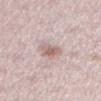Q: Was this lesion biopsied?
A: imaged on a skin check; not biopsied
Q: Where on the body is the lesion?
A: the left lower leg
Q: What kind of image is this?
A: ~15 mm tile from a whole-body skin photo
Q: Who is the patient?
A: female, aged around 30